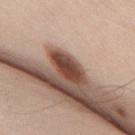No biopsy was performed on this lesion — it was imaged during a full skin examination and was not determined to be concerning.
A male patient aged approximately 65.
This is a white-light tile.
This image is a 15 mm lesion crop taken from a total-body photograph.
Measured at roughly 4.5 mm in maximum diameter.
The lesion-visualizer software estimated an average lesion color of about L≈43 a*≈22 b*≈26 (CIELAB), about 19 CIELAB-L* units darker than the surrounding skin, and a normalized border contrast of about 13.5. The software also gave a color-variation rating of about 5/10 and peripheral color asymmetry of about 2. The analysis additionally found a lesion-detection confidence of about 95/100.
From the upper back.